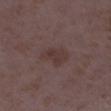• follow-up: catalogued during a skin exam; not biopsied
• site: the right lower leg
• tile lighting: white-light illumination
• acquisition: ~15 mm crop, total-body skin-cancer survey
• subject: female, aged 33 to 37
• image-analysis metrics: a lesion area of about 6.5 mm² and two-axis asymmetry of about 0.3; a mean CIELAB color near L≈35 a*≈16 b*≈18, a lesion–skin lightness drop of about 5, and a normalized lesion–skin contrast near 5.5